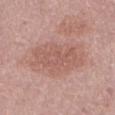Q: Is there a histopathology result?
A: imaged on a skin check; not biopsied
Q: Who is the patient?
A: male, in their mid- to late 70s
Q: Where on the body is the lesion?
A: the abdomen
Q: How large is the lesion?
A: ≈7 mm
Q: Automated lesion metrics?
A: a border-irregularity rating of about 3.5/10 and radial color variation of about 1; an automated nevus-likeness rating near 0 out of 100 and a lesion-detection confidence of about 100/100
Q: What kind of image is this?
A: 15 mm crop, total-body photography
Q: Illumination type?
A: white-light illumination